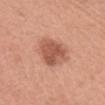{
  "biopsy_status": "not biopsied; imaged during a skin examination",
  "patient": {
    "sex": "female",
    "age_approx": 35
  },
  "site": "head or neck",
  "image": {
    "source": "total-body photography crop",
    "field_of_view_mm": 15
  },
  "automated_metrics": {
    "eccentricity": 0.6,
    "shape_asymmetry": 0.25,
    "cielab_L": 55,
    "cielab_a": 26,
    "cielab_b": 31,
    "vs_skin_darker_L": 12.0,
    "vs_skin_contrast_norm": 8.0
  },
  "lighting": "white-light",
  "lesion_size": {
    "long_diameter_mm_approx": 4.0
  }
}